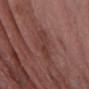Imaged during a routine full-body skin examination; the lesion was not biopsied and no histopathology is available. On the right forearm. Automated tile analysis of the lesion measured a lesion–skin lightness drop of about 6 and a normalized border contrast of about 5. The software also gave a border-irregularity rating of about 5/10. A male patient aged around 75. Approximately 4 mm at its widest. This image is a 15 mm lesion crop taken from a total-body photograph.